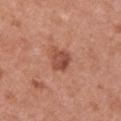Imaged during a routine full-body skin examination; the lesion was not biopsied and no histopathology is available.
A female subject roughly 40 years of age.
Imaged with white-light lighting.
Cropped from a total-body skin-imaging series; the visible field is about 15 mm.
Located on the left upper arm.
Automated image analysis of the tile measured border irregularity of about 3 on a 0–10 scale and peripheral color asymmetry of about 1.5.